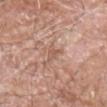biopsy_status: not biopsied; imaged during a skin examination
image:
  source: total-body photography crop
  field_of_view_mm: 15
site: left forearm
patient:
  sex: male
  age_approx: 60